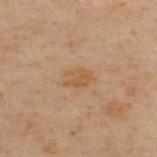Clinical impression: Captured during whole-body skin photography for melanoma surveillance; the lesion was not biopsied. Background: About 3.5 mm across. The tile uses cross-polarized illumination. From the upper back. The patient is a male roughly 50 years of age. Cropped from a whole-body photographic skin survey; the tile spans about 15 mm.